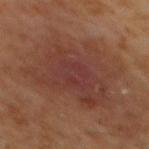This lesion was catalogued during total-body skin photography and was not selected for biopsy.
A 15 mm close-up tile from a total-body photography series done for melanoma screening.
The lesion is located on the mid back.
Captured under cross-polarized illumination.
Measured at roughly 9 mm in maximum diameter.
A male patient, about 60 years old.
The total-body-photography lesion software estimated a lesion area of about 44 mm² and a shape eccentricity near 0.6. The software also gave border irregularity of about 5.5 on a 0–10 scale, internal color variation of about 4 on a 0–10 scale, and peripheral color asymmetry of about 1.